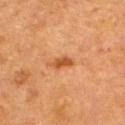Clinical impression:
No biopsy was performed on this lesion — it was imaged during a full skin examination and was not determined to be concerning.
Background:
A female subject aged 53 to 57. Measured at roughly 3 mm in maximum diameter. The lesion is on the upper back. The tile uses cross-polarized illumination. A 15 mm close-up extracted from a 3D total-body photography capture. Automated image analysis of the tile measured a border-irregularity rating of about 3/10 and internal color variation of about 1.5 on a 0–10 scale. The software also gave a detector confidence of about 100 out of 100 that the crop contains a lesion.